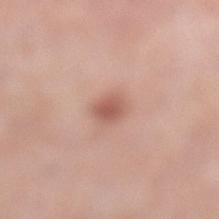workup=catalogued during a skin exam; not biopsied | patient=female, approximately 55 years of age | image=15 mm crop, total-body photography | lighting=white-light illumination | site=the left lower leg | automated metrics=an eccentricity of roughly 0.55 and two-axis asymmetry of about 0.2; a color-variation rating of about 2/10 and radial color variation of about 0.5 | diameter=about 2.5 mm.